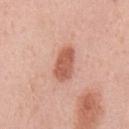Clinical impression:
The lesion was photographed on a routine skin check and not biopsied; there is no pathology result.
Background:
The lesion is on the front of the torso. A 15 mm close-up extracted from a 3D total-body photography capture. A male subject in their mid-50s. The lesion's longest dimension is about 4 mm. This is a white-light tile.